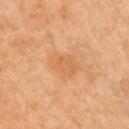Part of a total-body skin-imaging series; this lesion was reviewed on a skin check and was not flagged for biopsy.
Captured under cross-polarized illumination.
The lesion's longest dimension is about 3.5 mm.
A 15 mm close-up extracted from a 3D total-body photography capture.
A female subject, aged around 50.
From the right upper arm.